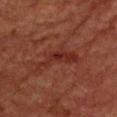Case summary:
– follow-up: imaged on a skin check; not biopsied